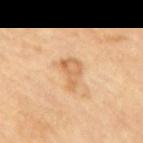Captured during whole-body skin photography for melanoma surveillance; the lesion was not biopsied. A close-up tile cropped from a whole-body skin photograph, about 15 mm across. Imaged with cross-polarized lighting. A male subject approximately 85 years of age or older. Approximately 3.5 mm at its widest. Located on the mid back.